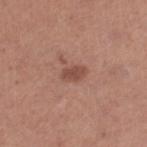No biopsy was performed on this lesion — it was imaged during a full skin examination and was not determined to be concerning.
Located on the right lower leg.
Approximately 2.5 mm at its widest.
A female subject, aged around 40.
Captured under white-light illumination.
Cropped from a total-body skin-imaging series; the visible field is about 15 mm.